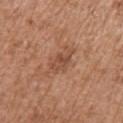notes: catalogued during a skin exam; not biopsied
acquisition: ~15 mm tile from a whole-body skin photo
subject: female, approximately 75 years of age
anatomic site: the right upper arm
illumination: white-light illumination